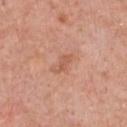Imaged during a routine full-body skin examination; the lesion was not biopsied and no histopathology is available. Captured under white-light illumination. An algorithmic analysis of the crop reported an area of roughly 3.5 mm², an eccentricity of roughly 0.9, and a shape-asymmetry score of about 0.3 (0 = symmetric). It also reported a lesion color around L≈58 a*≈25 b*≈32 in CIELAB. It also reported a border-irregularity index near 3.5/10 and internal color variation of about 0.5 on a 0–10 scale. The lesion is on the chest. A male subject aged 58–62. A region of skin cropped from a whole-body photographic capture, roughly 15 mm wide. Approximately 3 mm at its widest.